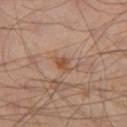Imaged during a routine full-body skin examination; the lesion was not biopsied and no histopathology is available. The recorded lesion diameter is about 2.5 mm. The lesion is located on the left lower leg. The tile uses cross-polarized illumination. A region of skin cropped from a whole-body photographic capture, roughly 15 mm wide. The subject is a male aged 43–47.